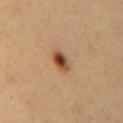Impression:
This lesion was catalogued during total-body skin photography and was not selected for biopsy.
Context:
From the mid back. A male subject, aged 48–52. A region of skin cropped from a whole-body photographic capture, roughly 15 mm wide. Measured at roughly 3 mm in maximum diameter.